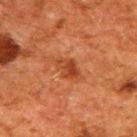Q: Patient demographics?
A: male, aged 58–62
Q: What is the imaging modality?
A: ~15 mm crop, total-body skin-cancer survey
Q: What is the anatomic site?
A: the upper back